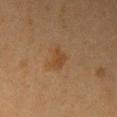Q: Is there a histopathology result?
A: imaged on a skin check; not biopsied
Q: Who is the patient?
A: female, roughly 40 years of age
Q: What lighting was used for the tile?
A: cross-polarized illumination
Q: What did automated image analysis measure?
A: an area of roughly 4.5 mm², a shape eccentricity near 0.7, and a shape-asymmetry score of about 0.3 (0 = symmetric); an average lesion color of about L≈38 a*≈17 b*≈31 (CIELAB), a lesion–skin lightness drop of about 6, and a normalized lesion–skin contrast near 6
Q: What is the anatomic site?
A: the right upper arm
Q: How was this image acquired?
A: ~15 mm tile from a whole-body skin photo